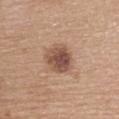Captured during whole-body skin photography for melanoma surveillance; the lesion was not biopsied.
On the back.
A female patient approximately 65 years of age.
A 15 mm close-up tile from a total-body photography series done for melanoma screening.